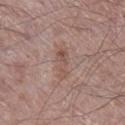The lesion was photographed on a routine skin check and not biopsied; there is no pathology result. The subject is a male aged 63 to 67. A close-up tile cropped from a whole-body skin photograph, about 15 mm across. Approximately 4.5 mm at its widest. From the right lower leg. This is a white-light tile.